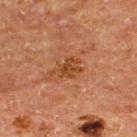This lesion was catalogued during total-body skin photography and was not selected for biopsy. Cropped from a total-body skin-imaging series; the visible field is about 15 mm. Imaged with cross-polarized lighting. The patient is a male approximately 65 years of age. The lesion is located on the upper back.